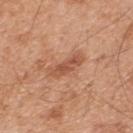| field | value |
|---|---|
| automated lesion analysis | a lesion area of about 6.5 mm² and an eccentricity of roughly 0.95; a lesion–skin lightness drop of about 9 and a lesion-to-skin contrast of about 6.5 (normalized; higher = more distinct) |
| tile lighting | white-light |
| image | ~15 mm crop, total-body skin-cancer survey |
| site | the upper back |
| subject | male, about 45 years old |